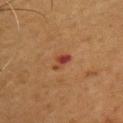Q: Is there a histopathology result?
A: no biopsy performed (imaged during a skin exam)
Q: What did automated image analysis measure?
A: a nevus-likeness score of about 0/100 and lesion-presence confidence of about 100/100
Q: What are the patient's age and sex?
A: male, aged 53 to 57
Q: What is the anatomic site?
A: the chest
Q: What kind of image is this?
A: total-body-photography crop, ~15 mm field of view